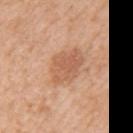The lesion was tiled from a total-body skin photograph and was not biopsied.